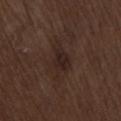Imaged during a routine full-body skin examination; the lesion was not biopsied and no histopathology is available. The lesion is located on the lower back. The lesion-visualizer software estimated a mean CIELAB color near L≈23 a*≈15 b*≈17 and a lesion-to-skin contrast of about 8 (normalized; higher = more distinct). It also reported a border-irregularity index near 4.5/10, a color-variation rating of about 2/10, and a peripheral color-asymmetry measure near 0.5. The patient is a male aged 68 to 72. Approximately 4.5 mm at its widest. A 15 mm crop from a total-body photograph taken for skin-cancer surveillance. The tile uses white-light illumination.